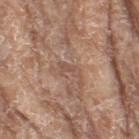Case summary:
– workup — catalogued during a skin exam; not biopsied
– lesion diameter — about 2.5 mm
– patient — female, aged 73–77
– site — the right thigh
– image — ~15 mm tile from a whole-body skin photo
– lighting — white-light